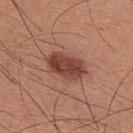Clinical impression:
No biopsy was performed on this lesion — it was imaged during a full skin examination and was not determined to be concerning.
Clinical summary:
Located on the upper back. A 15 mm crop from a total-body photograph taken for skin-cancer surveillance. The total-body-photography lesion software estimated an area of roughly 12 mm² and a shape-asymmetry score of about 0.1 (0 = symmetric). It also reported a within-lesion color-variation index near 4.5/10 and a peripheral color-asymmetry measure near 1.5. And it measured an automated nevus-likeness rating near 95 out of 100. Approximately 5.5 mm at its widest. The subject is a male aged approximately 35.